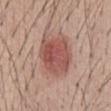The lesion was tiled from a total-body skin photograph and was not biopsied. A male subject, aged approximately 40. Approximately 6 mm at its widest. An algorithmic analysis of the crop reported an outline eccentricity of about 0.7 (0 = round, 1 = elongated) and a symmetry-axis asymmetry near 0.15. And it measured a mean CIELAB color near L≈52 a*≈24 b*≈25, a lesion–skin lightness drop of about 12, and a lesion-to-skin contrast of about 8 (normalized; higher = more distinct). And it measured a within-lesion color-variation index near 4.5/10 and a peripheral color-asymmetry measure near 1.5. The software also gave a nevus-likeness score of about 100/100 and a detector confidence of about 100 out of 100 that the crop contains a lesion. Imaged with white-light lighting. The lesion is on the abdomen. This image is a 15 mm lesion crop taken from a total-body photograph.